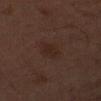<record>
  <biopsy_status>not biopsied; imaged during a skin examination</biopsy_status>
  <patient>
    <sex>male</sex>
    <age_approx>60</age_approx>
  </patient>
  <automated_metrics>
    <cielab_L>20</cielab_L>
    <cielab_a>14</cielab_a>
    <cielab_b>19</cielab_b>
    <vs_skin_darker_L>4.0</vs_skin_darker_L>
    <vs_skin_contrast_norm>6.0</vs_skin_contrast_norm>
  </automated_metrics>
  <site>right upper arm</site>
  <lesion_size>
    <long_diameter_mm_approx>3.0</long_diameter_mm_approx>
  </lesion_size>
  <image>
    <source>total-body photography crop</source>
    <field_of_view_mm>15</field_of_view_mm>
  </image>
  <lighting>cross-polarized</lighting>
</record>